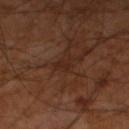Impression: Captured during whole-body skin photography for melanoma surveillance; the lesion was not biopsied. Clinical summary: A 15 mm close-up tile from a total-body photography series done for melanoma screening. The lesion is located on the left lower leg. Approximately 2.5 mm at its widest. A male subject, aged 58–62. Captured under cross-polarized illumination.